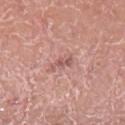follow-up: catalogued during a skin exam; not biopsied
illumination: white-light illumination
anatomic site: the left lower leg
imaging modality: ~15 mm tile from a whole-body skin photo
TBP lesion metrics: an outline eccentricity of about 0.85 (0 = round, 1 = elongated) and a symmetry-axis asymmetry near 0.4; an average lesion color of about L≈57 a*≈24 b*≈24 (CIELAB), a lesion–skin lightness drop of about 9, and a lesion-to-skin contrast of about 5.5 (normalized; higher = more distinct)
patient: male, about 50 years old